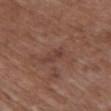The lesion was tiled from a total-body skin photograph and was not biopsied.
A region of skin cropped from a whole-body photographic capture, roughly 15 mm wide.
A female patient aged 78 to 82.
Imaged with white-light lighting.
The lesion is on the chest.